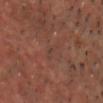The lesion was tiled from a total-body skin photograph and was not biopsied.
A male subject in their mid-50s.
The lesion is located on the head or neck.
Imaged with cross-polarized lighting.
This image is a 15 mm lesion crop taken from a total-body photograph.
The lesion-visualizer software estimated an area of roughly 2 mm² and an eccentricity of roughly 0.9. It also reported a lesion color around L≈37 a*≈19 b*≈22 in CIELAB, about 4 CIELAB-L* units darker than the surrounding skin, and a normalized border contrast of about 4.5.
Approximately 2.5 mm at its widest.